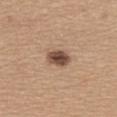Impression: Captured during whole-body skin photography for melanoma surveillance; the lesion was not biopsied. Clinical summary: A lesion tile, about 15 mm wide, cut from a 3D total-body photograph. The tile uses white-light illumination. A female patient, aged 58–62. The lesion is on the back. The total-body-photography lesion software estimated an average lesion color of about L≈48 a*≈18 b*≈27 (CIELAB) and roughly 16 lightness units darker than nearby skin.